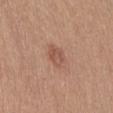{
  "biopsy_status": "not biopsied; imaged during a skin examination",
  "lighting": "white-light",
  "site": "front of the torso",
  "patient": {
    "sex": "female",
    "age_approx": 55
  },
  "automated_metrics": {
    "cielab_L": 53,
    "cielab_a": 22,
    "cielab_b": 29,
    "vs_skin_contrast_norm": 6.0
  },
  "image": {
    "source": "total-body photography crop",
    "field_of_view_mm": 15
  }
}